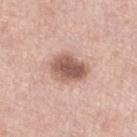Acquisition and patient details: A roughly 15 mm field-of-view crop from a total-body skin photograph. Longest diameter approximately 4.5 mm. Located on the left lower leg. A female patient, about 70 years old.